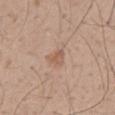Background: The tile uses white-light illumination. The total-body-photography lesion software estimated a lesion area of about 3.5 mm², an eccentricity of roughly 0.65, and a symmetry-axis asymmetry near 0.4. The analysis additionally found an average lesion color of about L≈57 a*≈19 b*≈29 (CIELAB) and a lesion–skin lightness drop of about 8. It also reported a border-irregularity index near 3.5/10, internal color variation of about 1.5 on a 0–10 scale, and peripheral color asymmetry of about 0.5. A roughly 15 mm field-of-view crop from a total-body skin photograph. The recorded lesion diameter is about 2.5 mm. A male patient aged approximately 50. From the mid back.